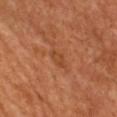biopsy_status: not biopsied; imaged during a skin examination
patient:
  sex: female
  age_approx: 65
image:
  source: total-body photography crop
  field_of_view_mm: 15
lighting: cross-polarized
lesion_size:
  long_diameter_mm_approx: 2.5
automated_metrics:
  cielab_L: 44
  cielab_a: 26
  cielab_b: 37
  vs_skin_darker_L: 6.0
  border_irregularity_0_10: 3.5
  color_variation_0_10: 0.5
  peripheral_color_asymmetry: 0.0
  nevus_likeness_0_100: 0
site: front of the torso